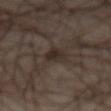- workup · imaged on a skin check; not biopsied
- subject · male, aged 63 to 67
- image · ~15 mm crop, total-body skin-cancer survey
- body site · the abdomen